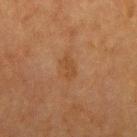Q: Was a biopsy performed?
A: imaged on a skin check; not biopsied
Q: Lesion location?
A: the arm
Q: What are the patient's age and sex?
A: female, in their mid-50s
Q: What kind of image is this?
A: ~15 mm tile from a whole-body skin photo
Q: How large is the lesion?
A: about 2.5 mm
Q: Illumination type?
A: cross-polarized illumination